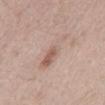notes: no biopsy performed (imaged during a skin exam) | patient: male, roughly 65 years of age | lighting: white-light illumination | imaging modality: ~15 mm tile from a whole-body skin photo | size: about 6 mm | automated lesion analysis: an area of roughly 11 mm² and a shape eccentricity near 0.95; an automated nevus-likeness rating near 0 out of 100 | site: the chest.